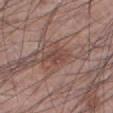Clinical impression: Imaged during a routine full-body skin examination; the lesion was not biopsied and no histopathology is available. Image and clinical context: Automated image analysis of the tile measured a lesion area of about 4.5 mm² and a shape eccentricity near 0.7. And it measured a lesion color around L≈45 a*≈20 b*≈22 in CIELAB, about 7 CIELAB-L* units darker than the surrounding skin, and a normalized lesion–skin contrast near 6. The software also gave a classifier nevus-likeness of about 25/100. A male patient aged 58 to 62. Imaged with white-light lighting. A region of skin cropped from a whole-body photographic capture, roughly 15 mm wide. On the left lower leg.